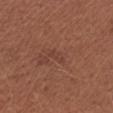Assessment:
This lesion was catalogued during total-body skin photography and was not selected for biopsy.
Image and clinical context:
Cropped from a whole-body photographic skin survey; the tile spans about 15 mm. The lesion is located on the right upper arm. A female subject, in their mid-50s. An algorithmic analysis of the crop reported an area of roughly 2.5 mm², an outline eccentricity of about 0.9 (0 = round, 1 = elongated), and two-axis asymmetry of about 0.35. The analysis additionally found a detector confidence of about 85 out of 100 that the crop contains a lesion.